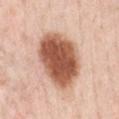biopsy status: imaged on a skin check; not biopsied
lighting: white-light illumination
size: ~7.5 mm (longest diameter)
automated metrics: a lesion area of about 30 mm², an outline eccentricity of about 0.7 (0 = round, 1 = elongated), and a symmetry-axis asymmetry near 0.15; a mean CIELAB color near L≈57 a*≈24 b*≈32 and a lesion–skin lightness drop of about 20; internal color variation of about 6 on a 0–10 scale and a peripheral color-asymmetry measure near 2
image: ~15 mm tile from a whole-body skin photo
location: the front of the torso
subject: male, in their 50s